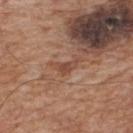workup = imaged on a skin check; not biopsied
site = the back
TBP lesion metrics = a mean CIELAB color near L≈47 a*≈22 b*≈29 and a normalized lesion–skin contrast near 6; border irregularity of about 5.5 on a 0–10 scale, internal color variation of about 1 on a 0–10 scale, and radial color variation of about 0.5
patient = male, roughly 65 years of age
diameter = ≈3 mm
image = total-body-photography crop, ~15 mm field of view